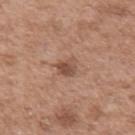Q: Was this lesion biopsied?
A: catalogued during a skin exam; not biopsied
Q: Patient demographics?
A: male, in their 50s
Q: What lighting was used for the tile?
A: white-light
Q: What did automated image analysis measure?
A: a mean CIELAB color near L≈50 a*≈20 b*≈28 and roughly 10 lightness units darker than nearby skin; a nevus-likeness score of about 50/100 and lesion-presence confidence of about 100/100
Q: Where on the body is the lesion?
A: the left upper arm
Q: How was this image acquired?
A: ~15 mm crop, total-body skin-cancer survey
Q: Lesion size?
A: ≈2.5 mm